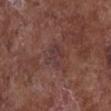Q: Was this lesion biopsied?
A: catalogued during a skin exam; not biopsied
Q: Lesion size?
A: about 3 mm
Q: What kind of image is this?
A: ~15 mm crop, total-body skin-cancer survey
Q: What are the patient's age and sex?
A: female, roughly 80 years of age
Q: Lesion location?
A: the chest